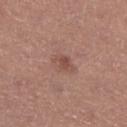Clinical impression: Recorded during total-body skin imaging; not selected for excision or biopsy.